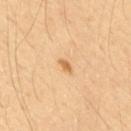notes: catalogued during a skin exam; not biopsied | body site: the upper back | patient: male, roughly 55 years of age | acquisition: ~15 mm tile from a whole-body skin photo.